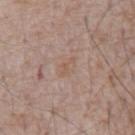  biopsy_status: not biopsied; imaged during a skin examination
  image:
    source: total-body photography crop
    field_of_view_mm: 15
  lighting: white-light
  site: abdomen
  patient:
    sex: male
    age_approx: 70
  lesion_size:
    long_diameter_mm_approx: 2.5